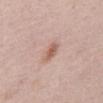Assessment: Imaged during a routine full-body skin examination; the lesion was not biopsied and no histopathology is available. Clinical summary: A male subject, about 65 years old. The tile uses white-light illumination. From the mid back. A roughly 15 mm field-of-view crop from a total-body skin photograph. Longest diameter approximately 2.5 mm. Automated image analysis of the tile measured an area of roughly 4 mm² and an outline eccentricity of about 0.8 (0 = round, 1 = elongated). It also reported an average lesion color of about L≈59 a*≈20 b*≈27 (CIELAB). The analysis additionally found a classifier nevus-likeness of about 65/100.